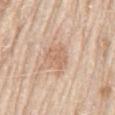Findings:
* biopsy status · total-body-photography surveillance lesion; no biopsy
* illumination · white-light
* imaging modality · total-body-photography crop, ~15 mm field of view
* lesion diameter · ~3 mm (longest diameter)
* patient · male, roughly 80 years of age
* TBP lesion metrics · an area of roughly 7 mm²; internal color variation of about 2 on a 0–10 scale and a peripheral color-asymmetry measure near 0.5; an automated nevus-likeness rating near 10 out of 100 and a detector confidence of about 100 out of 100 that the crop contains a lesion
* location · the mid back